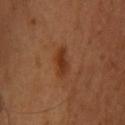  biopsy_status: not biopsied; imaged during a skin examination
  lighting: cross-polarized
  image:
    source: total-body photography crop
    field_of_view_mm: 15
  automated_metrics:
    border_irregularity_0_10: 4.0
    color_variation_0_10: 2.0
    peripheral_color_asymmetry: 0.5
  site: head or neck
  patient:
    sex: female
    age_approx: 35